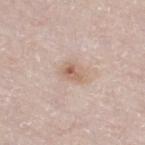  site: right thigh
  image:
    source: total-body photography crop
    field_of_view_mm: 15
  patient:
    sex: male
    age_approx: 80
  lesion_size:
    long_diameter_mm_approx: 3.0
  lighting: white-light